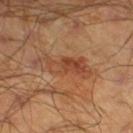Q: Is there a histopathology result?
A: total-body-photography surveillance lesion; no biopsy
Q: What is the imaging modality?
A: total-body-photography crop, ~15 mm field of view
Q: What is the anatomic site?
A: the left lower leg
Q: Who is the patient?
A: male, about 45 years old
Q: What lighting was used for the tile?
A: cross-polarized